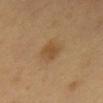Q: Who is the patient?
A: male, aged approximately 60
Q: Lesion location?
A: the abdomen
Q: How was the tile lit?
A: cross-polarized
Q: Automated lesion metrics?
A: a lesion area of about 4.5 mm², an eccentricity of roughly 0.55, and two-axis asymmetry of about 0.2; roughly 7 lightness units darker than nearby skin and a lesion-to-skin contrast of about 6 (normalized; higher = more distinct)
Q: What is the imaging modality?
A: ~15 mm tile from a whole-body skin photo
Q: What is the lesion's diameter?
A: ≈2.5 mm